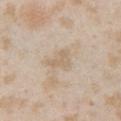Part of a total-body skin-imaging series; this lesion was reviewed on a skin check and was not flagged for biopsy.
Cropped from a total-body skin-imaging series; the visible field is about 15 mm.
Measured at roughly 3 mm in maximum diameter.
An algorithmic analysis of the crop reported an average lesion color of about L≈65 a*≈12 b*≈30 (CIELAB) and a lesion–skin lightness drop of about 7. The software also gave a border-irregularity rating of about 5/10 and peripheral color asymmetry of about 0.5. The software also gave a nevus-likeness score of about 0/100 and a lesion-detection confidence of about 100/100.
The lesion is on the left upper arm.
A female patient, in their mid- to late 20s.